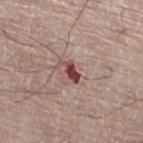Q: Is there a histopathology result?
A: no biopsy performed (imaged during a skin exam)
Q: What is the anatomic site?
A: the right leg
Q: Patient demographics?
A: male, about 80 years old
Q: What did automated image analysis measure?
A: internal color variation of about 0.5 on a 0–10 scale; a nevus-likeness score of about 35/100 and a detector confidence of about 100 out of 100 that the crop contains a lesion
Q: How was this image acquired?
A: ~15 mm tile from a whole-body skin photo